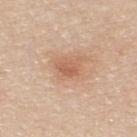workup: catalogued during a skin exam; not biopsied | image: ~15 mm tile from a whole-body skin photo | automated lesion analysis: a lesion area of about 4 mm², a shape eccentricity near 0.65, and a symmetry-axis asymmetry near 0.25; lesion-presence confidence of about 100/100 | subject: male, about 35 years old | site: the upper back.